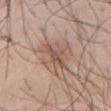tile lighting: white-light illumination | patient: male, roughly 30 years of age | body site: the front of the torso | image source: total-body-photography crop, ~15 mm field of view.